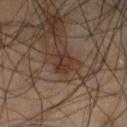No biopsy was performed on this lesion — it was imaged during a full skin examination and was not determined to be concerning.
On the left lower leg.
A male patient aged approximately 40.
Captured under cross-polarized illumination.
Cropped from a whole-body photographic skin survey; the tile spans about 15 mm.